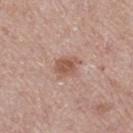Impression:
No biopsy was performed on this lesion — it was imaged during a full skin examination and was not determined to be concerning.
Acquisition and patient details:
The lesion's longest dimension is about 3 mm. From the leg. This is a white-light tile. This image is a 15 mm lesion crop taken from a total-body photograph. The patient is a female in their mid-60s.